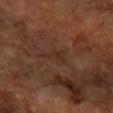A female patient, aged around 65. A close-up tile cropped from a whole-body skin photograph, about 15 mm across. The lesion is on the right forearm. An algorithmic analysis of the crop reported an average lesion color of about L≈28 a*≈19 b*≈24 (CIELAB) and a normalized lesion–skin contrast near 4.5. It also reported a nevus-likeness score of about 0/100 and a lesion-detection confidence of about 95/100.